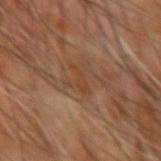{"biopsy_status": "not biopsied; imaged during a skin examination", "site": "right forearm", "lesion_size": {"long_diameter_mm_approx": 3.0}, "image": {"source": "total-body photography crop", "field_of_view_mm": 15}, "lighting": "cross-polarized", "patient": {"sex": "male", "age_approx": 65}}